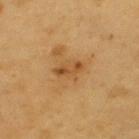{
  "biopsy_status": "not biopsied; imaged during a skin examination",
  "patient": {
    "sex": "male",
    "age_approx": 60
  },
  "lesion_size": {
    "long_diameter_mm_approx": 3.5
  },
  "lighting": "cross-polarized",
  "site": "upper back",
  "image": {
    "source": "total-body photography crop",
    "field_of_view_mm": 15
  },
  "automated_metrics": {
    "area_mm2_approx": 5.5,
    "eccentricity": 0.8,
    "shape_asymmetry": 0.3,
    "cielab_L": 51,
    "cielab_a": 21,
    "cielab_b": 41,
    "vs_skin_darker_L": 9.0,
    "vs_skin_contrast_norm": 6.5,
    "lesion_detection_confidence_0_100": 100
  }
}